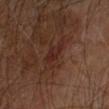biopsy_status: not biopsied; imaged during a skin examination